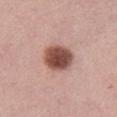Clinical impression: Recorded during total-body skin imaging; not selected for excision or biopsy. Clinical summary: A female patient, aged approximately 55. This image is a 15 mm lesion crop taken from a total-body photograph. On the leg. An algorithmic analysis of the crop reported a border-irregularity index near 1/10.